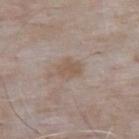| feature | finding |
|---|---|
| biopsy status | total-body-photography surveillance lesion; no biopsy |
| image | ~15 mm tile from a whole-body skin photo |
| subject | male, in their mid- to late 60s |
| TBP lesion metrics | border irregularity of about 2 on a 0–10 scale, internal color variation of about 1.5 on a 0–10 scale, and radial color variation of about 0.5; a nevus-likeness score of about 0/100 and lesion-presence confidence of about 100/100 |
| lighting | white-light illumination |
| body site | the back |
| diameter | about 3 mm |